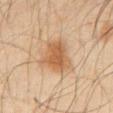The lesion was photographed on a routine skin check and not biopsied; there is no pathology result.